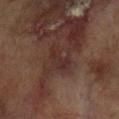biopsy status: total-body-photography surveillance lesion; no biopsy
subject: female, aged approximately 80
imaging modality: ~15 mm crop, total-body skin-cancer survey
location: the right arm
image-analysis metrics: a lesion area of about 5 mm² and a shape-asymmetry score of about 0.35 (0 = symmetric); an average lesion color of about L≈26 a*≈17 b*≈19 (CIELAB) and roughly 4 lightness units darker than nearby skin; a border-irregularity rating of about 4.5/10, internal color variation of about 1.5 on a 0–10 scale, and peripheral color asymmetry of about 0.5; lesion-presence confidence of about 95/100